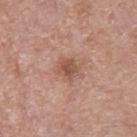workup = imaged on a skin check; not biopsied | subject = male, approximately 55 years of age | image source = total-body-photography crop, ~15 mm field of view | illumination = white-light illumination | diameter = ~2.5 mm (longest diameter).